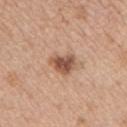follow-up = total-body-photography surveillance lesion; no biopsy | body site = the chest | image source = 15 mm crop, total-body photography | subject = female, about 45 years old | tile lighting = white-light illumination | lesion size = ~3.5 mm (longest diameter).